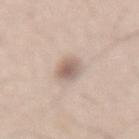Part of a total-body skin-imaging series; this lesion was reviewed on a skin check and was not flagged for biopsy. Located on the lower back. A 15 mm close-up extracted from a 3D total-body photography capture. Captured under white-light illumination. The total-body-photography lesion software estimated a shape eccentricity near 0.75 and a shape-asymmetry score of about 0.1 (0 = symmetric). The software also gave a lesion color around L≈61 a*≈15 b*≈24 in CIELAB and a lesion-to-skin contrast of about 7.5 (normalized; higher = more distinct). Approximately 3 mm at its widest. A male subject, about 60 years old.